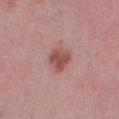notes: no biopsy performed (imaged during a skin exam)
diameter: ≈3 mm
tile lighting: white-light illumination
subject: female, aged approximately 45
image source: ~15 mm crop, total-body skin-cancer survey
body site: the right thigh
automated metrics: a footprint of about 6.5 mm² and two-axis asymmetry of about 0.2; a mean CIELAB color near L≈51 a*≈24 b*≈26, a lesion–skin lightness drop of about 11, and a normalized border contrast of about 8.5; a border-irregularity index near 2/10, a within-lesion color-variation index near 3/10, and radial color variation of about 1